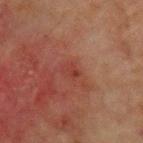{
  "biopsy_status": "not biopsied; imaged during a skin examination",
  "site": "chest",
  "image": {
    "source": "total-body photography crop",
    "field_of_view_mm": 15
  },
  "lighting": "cross-polarized",
  "patient": {
    "sex": "male",
    "age_approx": 65
  },
  "automated_metrics": {
    "area_mm2_approx": 3.0,
    "eccentricity": 0.75,
    "shape_asymmetry": 0.25,
    "cielab_L": 31,
    "cielab_a": 24,
    "cielab_b": 23,
    "vs_skin_darker_L": 5.0,
    "vs_skin_contrast_norm": 5.0,
    "nevus_likeness_0_100": 0,
    "lesion_detection_confidence_0_100": 100
  },
  "lesion_size": {
    "long_diameter_mm_approx": 2.0
  }
}